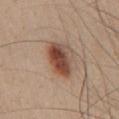Case summary:
– follow-up · no biopsy performed (imaged during a skin exam)
– image · ~15 mm tile from a whole-body skin photo
– lesion size · about 4.5 mm
– subject · male, aged approximately 60
– tile lighting · white-light illumination
– location · the chest
– automated metrics · a footprint of about 10 mm², an outline eccentricity of about 0.8 (0 = round, 1 = elongated), and a symmetry-axis asymmetry near 0.15; a lesion color around L≈46 a*≈21 b*≈28 in CIELAB, roughly 15 lightness units darker than nearby skin, and a normalized border contrast of about 11; a border-irregularity index near 2/10